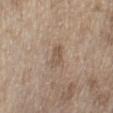follow-up: catalogued during a skin exam; not biopsied | patient: male, about 70 years old | location: the left lower leg | image: 15 mm crop, total-body photography | image-analysis metrics: an average lesion color of about L≈53 a*≈14 b*≈28 (CIELAB), about 8 CIELAB-L* units darker than the surrounding skin, and a normalized lesion–skin contrast near 6; border irregularity of about 2.5 on a 0–10 scale, a color-variation rating of about 2.5/10, and radial color variation of about 1 | tile lighting: white-light illumination | diameter: ~2.5 mm (longest diameter).